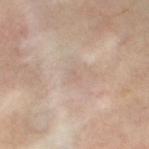The lesion was photographed on a routine skin check and not biopsied; there is no pathology result. A roughly 15 mm field-of-view crop from a total-body skin photograph. The lesion is on the left thigh. The tile uses cross-polarized illumination. A female subject, aged 58 to 62. The recorded lesion diameter is about 1 mm.